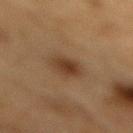Case summary:
- follow-up: catalogued during a skin exam; not biopsied